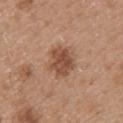The lesion was photographed on a routine skin check and not biopsied; there is no pathology result. The lesion is on the upper back. This image is a 15 mm lesion crop taken from a total-body photograph. A male patient, aged approximately 50. This is a white-light tile. The lesion's longest dimension is about 4 mm.